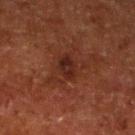This lesion was catalogued during total-body skin photography and was not selected for biopsy. A roughly 15 mm field-of-view crop from a total-body skin photograph. Longest diameter approximately 3 mm. The lesion-visualizer software estimated an outline eccentricity of about 0.65 (0 = round, 1 = elongated) and a symmetry-axis asymmetry near 0.2. It also reported a border-irregularity rating of about 2/10 and internal color variation of about 3 on a 0–10 scale. A male subject aged 78–82. Located on the leg.